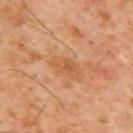This lesion was catalogued during total-body skin photography and was not selected for biopsy. A male subject, aged 58–62. The lesion is on the upper back. Cropped from a total-body skin-imaging series; the visible field is about 15 mm.